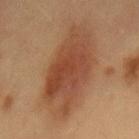{"biopsy_status": "not biopsied; imaged during a skin examination", "image": {"source": "total-body photography crop", "field_of_view_mm": 15}, "site": "back", "patient": {"sex": "female", "age_approx": 60}, "automated_metrics": {"area_mm2_approx": 32.0, "eccentricity": 0.85, "shape_asymmetry": 0.25, "nevus_likeness_0_100": 100, "lesion_detection_confidence_0_100": 100}, "lesion_size": {"long_diameter_mm_approx": 9.5}}